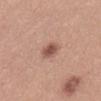The lesion was photographed on a routine skin check and not biopsied; there is no pathology result.